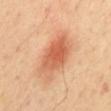| key | value |
|---|---|
| notes | imaged on a skin check; not biopsied |
| anatomic site | the mid back |
| acquisition | 15 mm crop, total-body photography |
| patient | male, roughly 50 years of age |